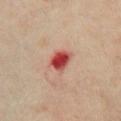follow-up — catalogued during a skin exam; not biopsied | anatomic site — the front of the torso | image source — 15 mm crop, total-body photography | tile lighting — cross-polarized | patient — female, aged approximately 45.